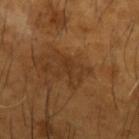Captured during whole-body skin photography for melanoma surveillance; the lesion was not biopsied. Longest diameter approximately 3 mm. On the left forearm. Imaged with cross-polarized lighting. A male subject in their mid- to late 60s. A lesion tile, about 15 mm wide, cut from a 3D total-body photograph.